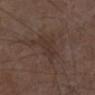Case summary:
* workup: no biopsy performed (imaged during a skin exam)
* site: the left lower leg
* image source: ~15 mm tile from a whole-body skin photo
* subject: male, aged approximately 75
* lesion size: about 4 mm
* image-analysis metrics: a footprint of about 7 mm², a shape eccentricity near 0.85, and two-axis asymmetry of about 0.35; a classifier nevus-likeness of about 0/100 and a lesion-detection confidence of about 90/100